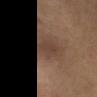Clinical impression: No biopsy was performed on this lesion — it was imaged during a full skin examination and was not determined to be concerning. Acquisition and patient details: Imaged with cross-polarized lighting. Located on the head or neck. The subject is a female aged 63 to 67. A roughly 15 mm field-of-view crop from a total-body skin photograph. Approximately 2.5 mm at its widest.